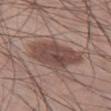No biopsy was performed on this lesion — it was imaged during a full skin examination and was not determined to be concerning. A 15 mm close-up tile from a total-body photography series done for melanoma screening. Automated tile analysis of the lesion measured a lesion area of about 20 mm², an eccentricity of roughly 0.8, and two-axis asymmetry of about 0.25. And it measured a lesion–skin lightness drop of about 11 and a lesion-to-skin contrast of about 8 (normalized; higher = more distinct). The software also gave an automated nevus-likeness rating near 45 out of 100 and a lesion-detection confidence of about 100/100. The subject is a male aged 58 to 62. On the left thigh. This is a white-light tile.